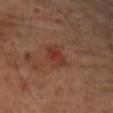Impression: Part of a total-body skin-imaging series; this lesion was reviewed on a skin check and was not flagged for biopsy. Acquisition and patient details: This is a cross-polarized tile. The recorded lesion diameter is about 3.5 mm. On the right forearm. Cropped from a whole-body photographic skin survey; the tile spans about 15 mm. The total-body-photography lesion software estimated an eccentricity of roughly 0.85. The software also gave a classifier nevus-likeness of about 5/100 and a lesion-detection confidence of about 100/100. A female subject, in their 60s.